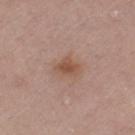Clinical impression:
No biopsy was performed on this lesion — it was imaged during a full skin examination and was not determined to be concerning.
Background:
This image is a 15 mm lesion crop taken from a total-body photograph. The patient is a male roughly 65 years of age. The lesion is located on the leg. Captured under white-light illumination. The lesion-visualizer software estimated an outline eccentricity of about 0.6 (0 = round, 1 = elongated). The analysis additionally found border irregularity of about 3 on a 0–10 scale, a within-lesion color-variation index near 2/10, and peripheral color asymmetry of about 0.5. It also reported a classifier nevus-likeness of about 65/100 and lesion-presence confidence of about 100/100. Measured at roughly 2.5 mm in maximum diameter.